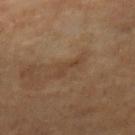Findings:
* subject — female, aged around 70
* acquisition — ~15 mm tile from a whole-body skin photo
* lighting — cross-polarized
* site — the right lower leg